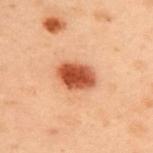* follow-up: total-body-photography surveillance lesion; no biopsy
* subject: male, in their mid-50s
* lighting: cross-polarized illumination
* site: the upper back
* size: ≈4 mm
* automated lesion analysis: an average lesion color of about L≈44 a*≈26 b*≈33 (CIELAB) and a lesion-to-skin contrast of about 11.5 (normalized; higher = more distinct); internal color variation of about 4.5 on a 0–10 scale and peripheral color asymmetry of about 1.5
* image: ~15 mm tile from a whole-body skin photo